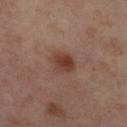Assessment:
Recorded during total-body skin imaging; not selected for excision or biopsy.
Image and clinical context:
A 15 mm crop from a total-body photograph taken for skin-cancer surveillance. A female patient aged around 55. From the right lower leg. An algorithmic analysis of the crop reported a footprint of about 6.5 mm², an outline eccentricity of about 0.7 (0 = round, 1 = elongated), and two-axis asymmetry of about 0.2. It also reported a lesion color around L≈41 a*≈21 b*≈27 in CIELAB, about 9 CIELAB-L* units darker than the surrounding skin, and a normalized lesion–skin contrast near 8.5. And it measured an automated nevus-likeness rating near 95 out of 100 and a detector confidence of about 100 out of 100 that the crop contains a lesion. This is a cross-polarized tile. Measured at roughly 3.5 mm in maximum diameter.